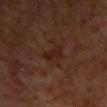The lesion was photographed on a routine skin check and not biopsied; there is no pathology result.
A 15 mm crop from a total-body photograph taken for skin-cancer surveillance.
On the upper back.
The patient is a male aged 58 to 62.